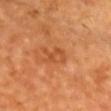- notes: no biopsy performed (imaged during a skin exam)
- lesion size: about 3 mm
- automated metrics: an outline eccentricity of about 0.65 (0 = round, 1 = elongated) and two-axis asymmetry of about 0.4; an average lesion color of about L≈51 a*≈29 b*≈42 (CIELAB) and a lesion-to-skin contrast of about 5 (normalized; higher = more distinct); border irregularity of about 4.5 on a 0–10 scale and radial color variation of about 1
- image source: total-body-photography crop, ~15 mm field of view
- body site: the chest
- patient: male, in their 70s